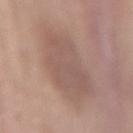Q: Was this lesion biopsied?
A: total-body-photography surveillance lesion; no biopsy
Q: What lighting was used for the tile?
A: white-light
Q: How was this image acquired?
A: ~15 mm crop, total-body skin-cancer survey
Q: Who is the patient?
A: female, aged 63–67
Q: What is the lesion's diameter?
A: about 6.5 mm
Q: What is the anatomic site?
A: the abdomen